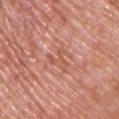No biopsy was performed on this lesion — it was imaged during a full skin examination and was not determined to be concerning. A 15 mm crop from a total-body photograph taken for skin-cancer surveillance. The subject is a male roughly 75 years of age. From the front of the torso.